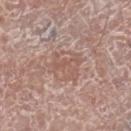Findings:
- notes — total-body-photography surveillance lesion; no biopsy